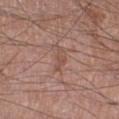{"biopsy_status": "not biopsied; imaged during a skin examination", "image": {"source": "total-body photography crop", "field_of_view_mm": 15}, "lesion_size": {"long_diameter_mm_approx": 3.0}, "lighting": "white-light", "site": "right lower leg", "patient": {"sex": "male", "age_approx": 55}}